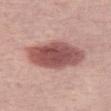Assessment:
Recorded during total-body skin imaging; not selected for excision or biopsy.
Acquisition and patient details:
This is a white-light tile. From the leg. Approximately 7 mm at its widest. The subject is a female approximately 65 years of age. A 15 mm close-up extracted from a 3D total-body photography capture. The lesion-visualizer software estimated a lesion area of about 25 mm², an eccentricity of roughly 0.8, and a shape-asymmetry score of about 0.15 (0 = symmetric). The analysis additionally found a lesion-to-skin contrast of about 10.5 (normalized; higher = more distinct). It also reported a border-irregularity index near 2/10, a within-lesion color-variation index near 4.5/10, and peripheral color asymmetry of about 1.5.